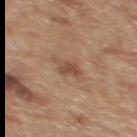Recorded during total-body skin imaging; not selected for excision or biopsy.
A male subject, aged approximately 60.
Approximately 3 mm at its widest.
Located on the upper back.
This image is a 15 mm lesion crop taken from a total-body photograph.
Captured under white-light illumination.